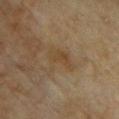{"biopsy_status": "not biopsied; imaged during a skin examination", "site": "front of the torso", "patient": {"sex": "female", "age_approx": 60}, "image": {"source": "total-body photography crop", "field_of_view_mm": 15}}